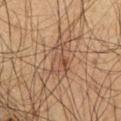workup: no biopsy performed (imaged during a skin exam) | site: the left thigh | image source: ~15 mm crop, total-body skin-cancer survey | lighting: cross-polarized illumination | patient: male, about 65 years old | automated lesion analysis: a lesion color around L≈41 a*≈16 b*≈26 in CIELAB and about 6 CIELAB-L* units darker than the surrounding skin; a classifier nevus-likeness of about 5/100 and a lesion-detection confidence of about 80/100 | diameter: ~4 mm (longest diameter).